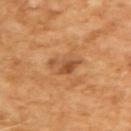A 15 mm close-up tile from a total-body photography series done for melanoma screening. The subject is a male about 65 years old. Captured under cross-polarized illumination. The lesion's longest dimension is about 4 mm. The lesion-visualizer software estimated an area of roughly 5 mm², an outline eccentricity of about 0.85 (0 = round, 1 = elongated), and a shape-asymmetry score of about 0.5 (0 = symmetric). It also reported peripheral color asymmetry of about 1.